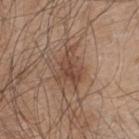image: ~15 mm tile from a whole-body skin photo
lesion size: ~5 mm (longest diameter)
location: the upper back
TBP lesion metrics: a border-irregularity index near 6/10, internal color variation of about 4 on a 0–10 scale, and a peripheral color-asymmetry measure near 1.5; an automated nevus-likeness rating near 85 out of 100 and lesion-presence confidence of about 100/100
patient: male, about 45 years old